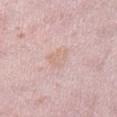Q: How was this image acquired?
A: 15 mm crop, total-body photography
Q: What are the patient's age and sex?
A: female, in their 40s
Q: Automated lesion metrics?
A: a border-irregularity index near 3/10, a color-variation rating of about 1.5/10, and peripheral color asymmetry of about 0.5
Q: How large is the lesion?
A: ~2.5 mm (longest diameter)
Q: What lighting was used for the tile?
A: white-light
Q: Lesion location?
A: the right lower leg